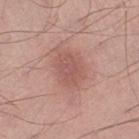Impression:
This lesion was catalogued during total-body skin photography and was not selected for biopsy.
Context:
An algorithmic analysis of the crop reported border irregularity of about 2 on a 0–10 scale, internal color variation of about 2.5 on a 0–10 scale, and a peripheral color-asymmetry measure near 1. The lesion is located on the right thigh. Captured under white-light illumination. The subject is a male in their mid- to late 50s. The lesion's longest dimension is about 4 mm. Cropped from a whole-body photographic skin survey; the tile spans about 15 mm.